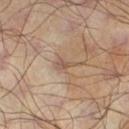Imaged during a routine full-body skin examination; the lesion was not biopsied and no histopathology is available. Approximately 2.5 mm at its widest. On the leg. A male subject, aged 63–67. A 15 mm crop from a total-body photograph taken for skin-cancer surveillance. An algorithmic analysis of the crop reported two-axis asymmetry of about 0.45. The analysis additionally found border irregularity of about 4.5 on a 0–10 scale, a color-variation rating of about 0.5/10, and peripheral color asymmetry of about 0. The software also gave a nevus-likeness score of about 0/100.